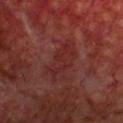Assessment: Recorded during total-body skin imaging; not selected for excision or biopsy. Clinical summary: A lesion tile, about 15 mm wide, cut from a 3D total-body photograph. Automated image analysis of the tile measured a lesion color around L≈28 a*≈27 b*≈22 in CIELAB, roughly 5 lightness units darker than nearby skin, and a normalized lesion–skin contrast near 5. And it measured border irregularity of about 4.5 on a 0–10 scale, a color-variation rating of about 3/10, and peripheral color asymmetry of about 1. The lesion is on the front of the torso. Approximately 6 mm at its widest. Imaged with cross-polarized lighting. The patient is a male about 70 years old.